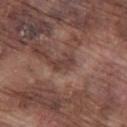The lesion is located on the left thigh.
The subject is a male aged 73–77.
Captured under white-light illumination.
Approximately 2.5 mm at its widest.
A 15 mm crop from a total-body photograph taken for skin-cancer surveillance.